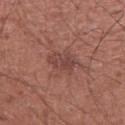workup: total-body-photography surveillance lesion; no biopsy
anatomic site: the left upper arm
subject: male, approximately 45 years of age
image source: total-body-photography crop, ~15 mm field of view
size: about 3.5 mm